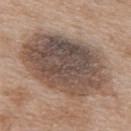Notes:
– biopsy status — imaged on a skin check; not biopsied
– patient — female, aged 38 to 42
– imaging modality — 15 mm crop, total-body photography
– diameter — ~10.5 mm (longest diameter)
– location — the back